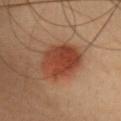Recorded during total-body skin imaging; not selected for excision or biopsy. The lesion's longest dimension is about 6 mm. Cropped from a whole-body photographic skin survey; the tile spans about 15 mm. An algorithmic analysis of the crop reported a lesion–skin lightness drop of about 10 and a lesion-to-skin contrast of about 9.5 (normalized; higher = more distinct). And it measured a border-irregularity rating of about 1.5/10, a within-lesion color-variation index near 4/10, and a peripheral color-asymmetry measure near 1.5. The subject is a female aged around 40. The lesion is on the head or neck.